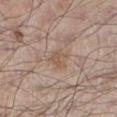The lesion's longest dimension is about 2.5 mm.
Imaged with white-light lighting.
The subject is a male aged approximately 55.
From the leg.
A lesion tile, about 15 mm wide, cut from a 3D total-body photograph.